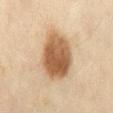  biopsy_status: not biopsied; imaged during a skin examination
  image:
    source: total-body photography crop
    field_of_view_mm: 15
  automated_metrics:
    area_mm2_approx: 21.0
    cielab_L: 50
    cielab_a: 17
    cielab_b: 32
    vs_skin_darker_L: 15.0
    vs_skin_contrast_norm: 10.5
    border_irregularity_0_10: 1.5
    color_variation_0_10: 5.0
    peripheral_color_asymmetry: 1.5
  lesion_size:
    long_diameter_mm_approx: 6.0
  patient:
    sex: female
    age_approx: 60
  site: mid back